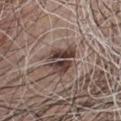The lesion was photographed on a routine skin check and not biopsied; there is no pathology result.
A male subject, approximately 65 years of age.
From the front of the torso.
The lesion-visualizer software estimated an area of roughly 11 mm², an eccentricity of roughly 0.65, and a symmetry-axis asymmetry near 0.25.
Cropped from a total-body skin-imaging series; the visible field is about 15 mm.